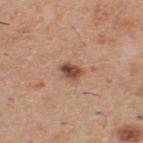workup: imaged on a skin check; not biopsied.